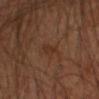This lesion was catalogued during total-body skin photography and was not selected for biopsy.
A male patient, in their mid- to late 40s.
From the arm.
The lesion's longest dimension is about 2.5 mm.
The tile uses cross-polarized illumination.
An algorithmic analysis of the crop reported an area of roughly 2.5 mm², a shape eccentricity near 0.9, and two-axis asymmetry of about 0.25. The analysis additionally found an average lesion color of about L≈28 a*≈19 b*≈26 (CIELAB), about 5 CIELAB-L* units darker than the surrounding skin, and a normalized border contrast of about 6. It also reported internal color variation of about 0 on a 0–10 scale and peripheral color asymmetry of about 0. The software also gave a classifier nevus-likeness of about 0/100 and lesion-presence confidence of about 100/100.
Cropped from a whole-body photographic skin survey; the tile spans about 15 mm.